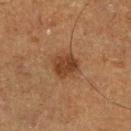Clinical impression:
Part of a total-body skin-imaging series; this lesion was reviewed on a skin check and was not flagged for biopsy.
Image and clinical context:
A roughly 15 mm field-of-view crop from a total-body skin photograph. The patient is a male in their mid- to late 70s. On the right lower leg.